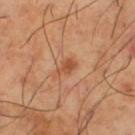workup = total-body-photography surveillance lesion; no biopsy | subject = male, aged approximately 65 | illumination = cross-polarized | imaging modality = total-body-photography crop, ~15 mm field of view | anatomic site = the left thigh.